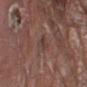Notes:
– biopsy status: imaged on a skin check; not biopsied
– anatomic site: the left lower leg
– tile lighting: white-light
– size: about 3 mm
– image: ~15 mm tile from a whole-body skin photo
– patient: male, aged approximately 80